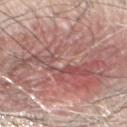Q: Was a biopsy performed?
A: imaged on a skin check; not biopsied
Q: How large is the lesion?
A: ≈14.5 mm
Q: What is the anatomic site?
A: the front of the torso
Q: What kind of image is this?
A: total-body-photography crop, ~15 mm field of view
Q: What lighting was used for the tile?
A: white-light illumination
Q: Who is the patient?
A: male, in their 80s